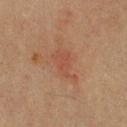Captured under cross-polarized illumination.
Cropped from a total-body skin-imaging series; the visible field is about 15 mm.
On the chest.
The lesion's longest dimension is about 4.5 mm.
Automated image analysis of the tile measured border irregularity of about 5.5 on a 0–10 scale and a within-lesion color-variation index near 1.5/10.
A male patient, aged 38–42.